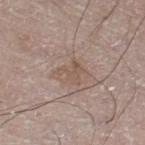| key | value |
|---|---|
| notes | total-body-photography surveillance lesion; no biopsy |
| body site | the left leg |
| lighting | white-light |
| TBP lesion metrics | a mean CIELAB color near L≈53 a*≈15 b*≈24, about 6 CIELAB-L* units darker than the surrounding skin, and a lesion-to-skin contrast of about 5 (normalized; higher = more distinct); internal color variation of about 2 on a 0–10 scale and peripheral color asymmetry of about 1; an automated nevus-likeness rating near 5 out of 100 and lesion-presence confidence of about 100/100 |
| patient | male, aged around 70 |
| imaging modality | total-body-photography crop, ~15 mm field of view |
| size | ~3 mm (longest diameter) |